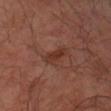Impression: Part of a total-body skin-imaging series; this lesion was reviewed on a skin check and was not flagged for biopsy. Background: Captured under cross-polarized illumination. A 15 mm crop from a total-body photograph taken for skin-cancer surveillance. The recorded lesion diameter is about 3 mm. The lesion is on the left arm. An algorithmic analysis of the crop reported a border-irregularity rating of about 2/10, a within-lesion color-variation index near 2.5/10, and peripheral color asymmetry of about 1. A male subject, roughly 50 years of age.